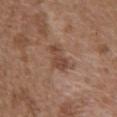Clinical impression:
The lesion was photographed on a routine skin check and not biopsied; there is no pathology result.
Background:
The lesion's longest dimension is about 4 mm. Cropped from a total-body skin-imaging series; the visible field is about 15 mm. The lesion is on the abdomen. The patient is a male in their mid- to late 70s. Imaged with white-light lighting.